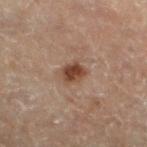| key | value |
|---|---|
| biopsy status | no biopsy performed (imaged during a skin exam) |
| lesion size | ~3 mm (longest diameter) |
| tile lighting | cross-polarized |
| body site | the left lower leg |
| patient | male, aged around 70 |
| image | ~15 mm crop, total-body skin-cancer survey |
| image-analysis metrics | a shape eccentricity near 0.5 and two-axis asymmetry of about 0.2; a border-irregularity index near 2/10, internal color variation of about 2.5 on a 0–10 scale, and radial color variation of about 1; a lesion-detection confidence of about 100/100 |